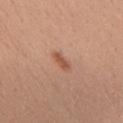Q: Is there a histopathology result?
A: no biopsy performed (imaged during a skin exam)
Q: Who is the patient?
A: female, aged 38 to 42
Q: What kind of image is this?
A: ~15 mm tile from a whole-body skin photo
Q: What is the anatomic site?
A: the back